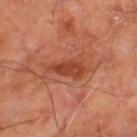This lesion was catalogued during total-body skin photography and was not selected for biopsy. Imaged with cross-polarized lighting. A patient approximately 65 years of age. Automated tile analysis of the lesion measured a lesion color around L≈45 a*≈30 b*≈34 in CIELAB and a lesion-to-skin contrast of about 7 (normalized; higher = more distinct). And it measured a border-irregularity rating of about 5/10 and peripheral color asymmetry of about 1. The lesion's longest dimension is about 5 mm. From the left thigh. A 15 mm close-up extracted from a 3D total-body photography capture.